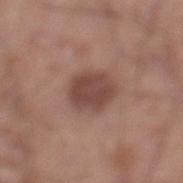Background: Measured at roughly 4.5 mm in maximum diameter. The lesion-visualizer software estimated an area of roughly 12 mm² and a symmetry-axis asymmetry near 0.15. It also reported a mean CIELAB color near L≈45 a*≈21 b*≈24, roughly 11 lightness units darker than nearby skin, and a normalized border contrast of about 8.5. And it measured a nevus-likeness score of about 65/100 and lesion-presence confidence of about 100/100. A 15 mm close-up extracted from a 3D total-body photography capture. On the left lower leg. A male patient, aged around 20. This is a white-light tile.